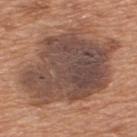No biopsy was performed on this lesion — it was imaged during a full skin examination and was not determined to be concerning. A male subject, in their mid- to late 60s. On the upper back. A 15 mm crop from a total-body photograph taken for skin-cancer surveillance.